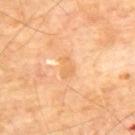Case summary:
– biopsy status · imaged on a skin check; not biopsied
– automated metrics · a footprint of about 3.5 mm², an outline eccentricity of about 0.8 (0 = round, 1 = elongated), and two-axis asymmetry of about 0.25; internal color variation of about 1 on a 0–10 scale and a peripheral color-asymmetry measure near 0.5; lesion-presence confidence of about 100/100
– subject · male, aged 68 to 72
– size · about 2.5 mm
– imaging modality · 15 mm crop, total-body photography
– location · the mid back